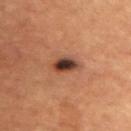Acquisition and patient details:
Measured at roughly 3.5 mm in maximum diameter. The lesion is located on the upper back. Cropped from a whole-body photographic skin survey; the tile spans about 15 mm. Captured under cross-polarized illumination. Automated tile analysis of the lesion measured an area of roughly 6 mm² and an eccentricity of roughly 0.8. And it measured a border-irregularity rating of about 2.5/10, a color-variation rating of about 7.5/10, and a peripheral color-asymmetry measure near 2. A female subject, roughly 80 years of age.